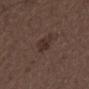Part of a total-body skin-imaging series; this lesion was reviewed on a skin check and was not flagged for biopsy.
An algorithmic analysis of the crop reported an average lesion color of about L≈30 a*≈15 b*≈19 (CIELAB), roughly 7 lightness units darker than nearby skin, and a lesion-to-skin contrast of about 7 (normalized; higher = more distinct).
The lesion is located on the left lower leg.
A roughly 15 mm field-of-view crop from a total-body skin photograph.
The recorded lesion diameter is about 3 mm.
A male subject, roughly 50 years of age.